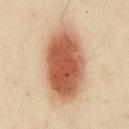The lesion was photographed on a routine skin check and not biopsied; there is no pathology result. A 15 mm close-up extracted from a 3D total-body photography capture. A male patient, approximately 50 years of age. The lesion is located on the chest. Measured at roughly 9.5 mm in maximum diameter.